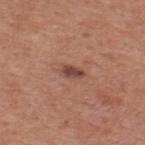follow-up: imaged on a skin check; not biopsied
subject: male, in their mid-50s
tile lighting: white-light
acquisition: ~15 mm tile from a whole-body skin photo
body site: the upper back